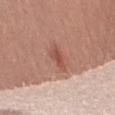Acquisition and patient details: A female subject aged around 20. Approximately 3 mm at its widest. The lesion is located on the left thigh. A close-up tile cropped from a whole-body skin photograph, about 15 mm across.